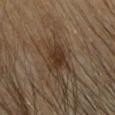Findings:
* biopsy status · imaged on a skin check; not biopsied
* body site · the head or neck
* lesion size · ≈3.5 mm
* patient · female, aged approximately 35
* image-analysis metrics · a border-irregularity rating of about 3.5/10, a within-lesion color-variation index near 3/10, and a peripheral color-asymmetry measure near 1; an automated nevus-likeness rating near 85 out of 100 and lesion-presence confidence of about 100/100
* image · total-body-photography crop, ~15 mm field of view